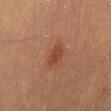The subject is a male aged approximately 55.
On the abdomen.
Cropped from a whole-body photographic skin survey; the tile spans about 15 mm.